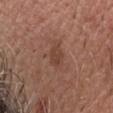Findings:
* notes — catalogued during a skin exam; not biopsied
* site — the head or neck
* acquisition — 15 mm crop, total-body photography
* subject — male, aged approximately 55
* tile lighting — white-light